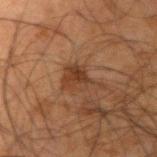The lesion was photographed on a routine skin check and not biopsied; there is no pathology result. A roughly 15 mm field-of-view crop from a total-body skin photograph. The lesion's longest dimension is about 4.5 mm. A male patient about 65 years old. The lesion is located on the arm.